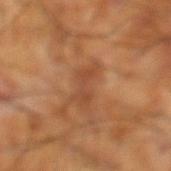A close-up tile cropped from a whole-body skin photograph, about 15 mm across.
On the left lower leg.
A male subject roughly 60 years of age.
About 4 mm across.
The total-body-photography lesion software estimated a border-irregularity index near 5.5/10 and a peripheral color-asymmetry measure near 0.5. And it measured a nevus-likeness score of about 0/100 and a lesion-detection confidence of about 100/100.